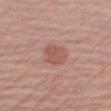Part of a total-body skin-imaging series; this lesion was reviewed on a skin check and was not flagged for biopsy. The tile uses white-light illumination. The lesion is located on the arm. Longest diameter approximately 3 mm. A close-up tile cropped from a whole-body skin photograph, about 15 mm across. The patient is a male aged around 60.